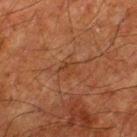Q: Is there a histopathology result?
A: imaged on a skin check; not biopsied
Q: What are the patient's age and sex?
A: male, aged around 80
Q: What is the anatomic site?
A: the leg
Q: How was this image acquired?
A: ~15 mm crop, total-body skin-cancer survey
Q: Illumination type?
A: cross-polarized
Q: How large is the lesion?
A: about 2.5 mm